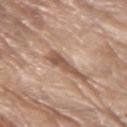<record>
<biopsy_status>not biopsied; imaged during a skin examination</biopsy_status>
<patient>
  <sex>male</sex>
  <age_approx>80</age_approx>
</patient>
<automated_metrics>
  <area_mm2_approx>4.0</area_mm2_approx>
  <cielab_L>53</cielab_L>
  <cielab_a>20</cielab_a>
  <cielab_b>29</cielab_b>
  <vs_skin_contrast_norm>9.0</vs_skin_contrast_norm>
  <nevus_likeness_0_100>5</nevus_likeness_0_100>
  <lesion_detection_confidence_0_100>80</lesion_detection_confidence_0_100>
</automated_metrics>
<site>right upper arm</site>
<lighting>white-light</lighting>
<lesion_size>
  <long_diameter_mm_approx>4.0</long_diameter_mm_approx>
</lesion_size>
<image>
  <source>total-body photography crop</source>
  <field_of_view_mm>15</field_of_view_mm>
</image>
</record>